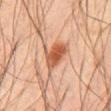Part of a total-body skin-imaging series; this lesion was reviewed on a skin check and was not flagged for biopsy.
A roughly 15 mm field-of-view crop from a total-body skin photograph.
On the lower back.
Imaged with cross-polarized lighting.
Approximately 3.5 mm at its widest.
The subject is a male roughly 70 years of age.